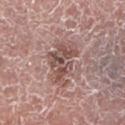From the left lower leg.
A male patient, about 75 years old.
Cropped from a total-body skin-imaging series; the visible field is about 15 mm.